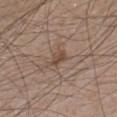{"biopsy_status": "not biopsied; imaged during a skin examination", "site": "left lower leg", "patient": {"sex": "male", "age_approx": 45}, "automated_metrics": {"peripheral_color_asymmetry": 1.0}, "lighting": "white-light", "image": {"source": "total-body photography crop", "field_of_view_mm": 15}}